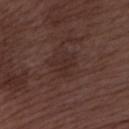This lesion was catalogued during total-body skin photography and was not selected for biopsy. The lesion-visualizer software estimated a mean CIELAB color near L≈28 a*≈17 b*≈19, about 4 CIELAB-L* units darker than the surrounding skin, and a normalized border contrast of about 5. And it measured a border-irregularity index near 5.5/10, internal color variation of about 1.5 on a 0–10 scale, and radial color variation of about 0.5. And it measured a classifier nevus-likeness of about 0/100 and a detector confidence of about 100 out of 100 that the crop contains a lesion. Approximately 3.5 mm at its widest. The tile uses white-light illumination. A male subject, about 75 years old. A roughly 15 mm field-of-view crop from a total-body skin photograph. On the left forearm.